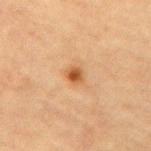Recorded during total-body skin imaging; not selected for excision or biopsy.
The lesion-visualizer software estimated a shape eccentricity near 0.55 and two-axis asymmetry of about 0.25. The software also gave radial color variation of about 0.5.
The recorded lesion diameter is about 2 mm.
A lesion tile, about 15 mm wide, cut from a 3D total-body photograph.
The tile uses cross-polarized illumination.
From the right upper arm.
A female patient, in their mid-60s.